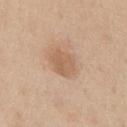biopsy_status: not biopsied; imaged during a skin examination
automated_metrics:
  area_mm2_approx: 7.0
  eccentricity: 0.6
  shape_asymmetry: 0.2
  cielab_L: 60
  cielab_a: 18
  cielab_b: 33
  vs_skin_darker_L: 9.0
  vs_skin_contrast_norm: 6.0
  nevus_likeness_0_100: 30
  lesion_detection_confidence_0_100: 100
image:
  source: total-body photography crop
  field_of_view_mm: 15
patient:
  sex: male
  age_approx: 60
site: chest
lighting: white-light